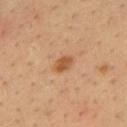Part of a total-body skin-imaging series; this lesion was reviewed on a skin check and was not flagged for biopsy.
The subject is a male about 35 years old.
An algorithmic analysis of the crop reported an area of roughly 4 mm², an eccentricity of roughly 0.75, and a shape-asymmetry score of about 0.2 (0 = symmetric). And it measured a classifier nevus-likeness of about 90/100 and a lesion-detection confidence of about 100/100.
From the back.
Cropped from a total-body skin-imaging series; the visible field is about 15 mm.
Approximately 2.5 mm at its widest.